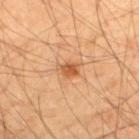  biopsy_status: not biopsied; imaged during a skin examination
  automated_metrics:
    border_irregularity_0_10: 2.5
    nevus_likeness_0_100: 85
    lesion_detection_confidence_0_100: 100
  image:
    source: total-body photography crop
    field_of_view_mm: 15
  site: back
  lesion_size:
    long_diameter_mm_approx: 2.5
  patient:
    sex: male
    age_approx: 55